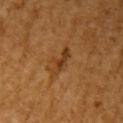Assessment:
Part of a total-body skin-imaging series; this lesion was reviewed on a skin check and was not flagged for biopsy.
Acquisition and patient details:
The tile uses cross-polarized illumination. Automated tile analysis of the lesion measured a lesion area of about 5 mm², a shape eccentricity near 0.9, and a shape-asymmetry score of about 0.35 (0 = symmetric). The software also gave a lesion color around L≈40 a*≈22 b*≈39 in CIELAB. The software also gave radial color variation of about 0.5. A lesion tile, about 15 mm wide, cut from a 3D total-body photograph. A female patient in their mid- to late 50s. About 4 mm across. The lesion is on the right upper arm.